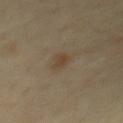The lesion was tiled from a total-body skin photograph and was not biopsied.